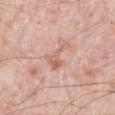Automated image analysis of the tile measured an area of roughly 6 mm² and two-axis asymmetry of about 0.65. It also reported a border-irregularity index near 7.5/10, a within-lesion color-variation index near 4/10, and a peripheral color-asymmetry measure near 1. The analysis additionally found a nevus-likeness score of about 0/100 and a detector confidence of about 100 out of 100 that the crop contains a lesion. The lesion is located on the abdomen. A male patient aged 78 to 82. This is a white-light tile. Measured at roughly 4.5 mm in maximum diameter. Cropped from a whole-body photographic skin survey; the tile spans about 15 mm.